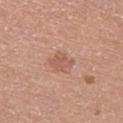Part of a total-body skin-imaging series; this lesion was reviewed on a skin check and was not flagged for biopsy.
A lesion tile, about 15 mm wide, cut from a 3D total-body photograph.
On the left thigh.
A female subject, aged 48–52.